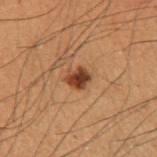This lesion was catalogued during total-body skin photography and was not selected for biopsy. From the right upper arm. A region of skin cropped from a whole-body photographic capture, roughly 15 mm wide. The lesion's longest dimension is about 2.5 mm. Imaged with cross-polarized lighting. A male patient in their 50s.